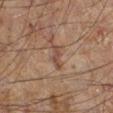Captured during whole-body skin photography for melanoma surveillance; the lesion was not biopsied.
A male patient, aged around 60.
The lesion is on the left leg.
A lesion tile, about 15 mm wide, cut from a 3D total-body photograph.